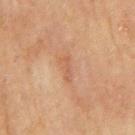Q: Was this lesion biopsied?
A: catalogued during a skin exam; not biopsied
Q: Where on the body is the lesion?
A: the back
Q: What are the patient's age and sex?
A: male, in their 70s
Q: Automated lesion metrics?
A: a classifier nevus-likeness of about 0/100 and lesion-presence confidence of about 100/100
Q: How was this image acquired?
A: 15 mm crop, total-body photography
Q: How large is the lesion?
A: ≈3 mm
Q: How was the tile lit?
A: cross-polarized